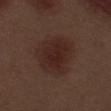Clinical summary:
The recorded lesion diameter is about 5.5 mm. Captured under white-light illumination. A 15 mm crop from a total-body photograph taken for skin-cancer surveillance. The lesion is on the left thigh. A male patient about 70 years old.